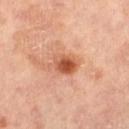This lesion was catalogued during total-body skin photography and was not selected for biopsy. A female patient, in their mid- to late 60s. The lesion is located on the leg. Longest diameter approximately 4 mm. A close-up tile cropped from a whole-body skin photograph, about 15 mm across. Imaged with cross-polarized lighting. Automated tile analysis of the lesion measured a footprint of about 7 mm² and a shape eccentricity near 0.75. And it measured a lesion color around L≈55 a*≈27 b*≈35 in CIELAB and a normalized lesion–skin contrast near 8.5. The analysis additionally found a border-irregularity rating of about 2.5/10, a color-variation rating of about 7/10, and a peripheral color-asymmetry measure near 2.